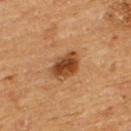Q: Was this lesion biopsied?
A: imaged on a skin check; not biopsied